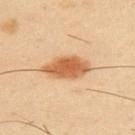Notes:
* workup · imaged on a skin check; not biopsied
* location · the upper back
* patient · male, in their 40s
* acquisition · ~15 mm tile from a whole-body skin photo
* diameter · about 6 mm
* tile lighting · cross-polarized illumination